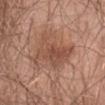{"lesion_size": {"long_diameter_mm_approx": 5.5}, "patient": {"sex": "male", "age_approx": 65}, "image": {"source": "total-body photography crop", "field_of_view_mm": 15}, "lighting": "white-light", "site": "abdomen"}